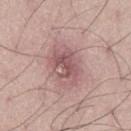notes=imaged on a skin check; not biopsied | anatomic site=the leg | subject=male, in their mid-50s | imaging modality=~15 mm tile from a whole-body skin photo.